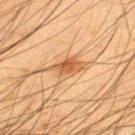follow-up: imaged on a skin check; not biopsied | subject: male, about 35 years old | image source: ~15 mm crop, total-body skin-cancer survey | location: the leg.